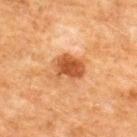Part of a total-body skin-imaging series; this lesion was reviewed on a skin check and was not flagged for biopsy.
A 15 mm close-up tile from a total-body photography series done for melanoma screening.
The subject is a male roughly 50 years of age.
This is a cross-polarized tile.
The lesion is located on the upper back.